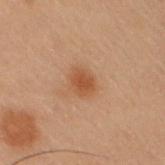biopsy status = no biopsy performed (imaged during a skin exam); diameter = ≈3 mm; subject = male, roughly 55 years of age; lighting = cross-polarized; acquisition = ~15 mm crop, total-body skin-cancer survey; anatomic site = the right upper arm.